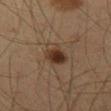Notes:
* biopsy status · imaged on a skin check; not biopsied
* patient · male, in their 60s
* body site · the leg
* acquisition · total-body-photography crop, ~15 mm field of view